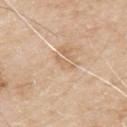Findings:
- biopsy status · catalogued during a skin exam; not biopsied
- anatomic site · the back
- lesion diameter · ≈3 mm
- lighting · white-light illumination
- imaging modality · ~15 mm tile from a whole-body skin photo
- subject · male, aged 78–82